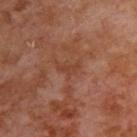<case>
<biopsy_status>not biopsied; imaged during a skin examination</biopsy_status>
<site>upper back</site>
<lighting>cross-polarized</lighting>
<patient>
  <sex>male</sex>
  <age_approx>70</age_approx>
</patient>
<lesion_size>
  <long_diameter_mm_approx>2.5</long_diameter_mm_approx>
</lesion_size>
<automated_metrics>
  <area_mm2_approx>3.0</area_mm2_approx>
  <eccentricity>0.85</eccentricity>
  <shape_asymmetry>0.45</shape_asymmetry>
  <vs_skin_darker_L>5.0</vs_skin_darker_L>
  <vs_skin_contrast_norm>5.0</vs_skin_contrast_norm>
  <nevus_likeness_0_100>0</nevus_likeness_0_100>
  <lesion_detection_confidence_0_100>100</lesion_detection_confidence_0_100>
</automated_metrics>
<image>
  <source>total-body photography crop</source>
  <field_of_view_mm>15</field_of_view_mm>
</image>
</case>